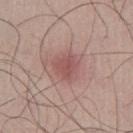Case summary:
* workup · imaged on a skin check; not biopsied
* size · ≈3 mm
* imaging modality · ~15 mm tile from a whole-body skin photo
* patient · male, roughly 50 years of age
* site · the left thigh